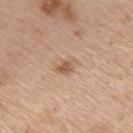Q: Was this lesion biopsied?
A: no biopsy performed (imaged during a skin exam)
Q: Automated lesion metrics?
A: an outline eccentricity of about 0.75 (0 = round, 1 = elongated) and two-axis asymmetry of about 0.45; an average lesion color of about L≈57 a*≈19 b*≈32 (CIELAB), about 10 CIELAB-L* units darker than the surrounding skin, and a lesion-to-skin contrast of about 7 (normalized; higher = more distinct)
Q: Patient demographics?
A: male, aged approximately 60
Q: What is the lesion's diameter?
A: about 2.5 mm
Q: What is the imaging modality?
A: ~15 mm tile from a whole-body skin photo
Q: Lesion location?
A: the left upper arm
Q: Illumination type?
A: white-light illumination